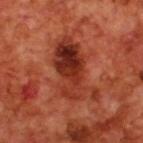{"biopsy_status": "not biopsied; imaged during a skin examination"}